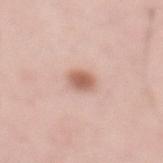No biopsy was performed on this lesion — it was imaged during a full skin examination and was not determined to be concerning. The subject is a male aged 58–62. Approximately 2.5 mm at its widest. Imaged with white-light lighting. A roughly 15 mm field-of-view crop from a total-body skin photograph. On the abdomen.